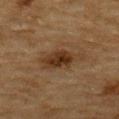Q: Is there a histopathology result?
A: no biopsy performed (imaged during a skin exam)
Q: What is the lesion's diameter?
A: about 5.5 mm
Q: How was this image acquired?
A: ~15 mm crop, total-body skin-cancer survey
Q: What are the patient's age and sex?
A: male, aged 83 or older
Q: Lesion location?
A: the upper back